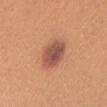A female patient approximately 25 years of age.
Captured under white-light illumination.
Cropped from a whole-body photographic skin survey; the tile spans about 15 mm.
An algorithmic analysis of the crop reported an area of roughly 11 mm², an eccentricity of roughly 0.8, and a symmetry-axis asymmetry near 0.1.
On the head or neck.
The recorded lesion diameter is about 5 mm.